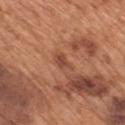  biopsy_status: not biopsied; imaged during a skin examination